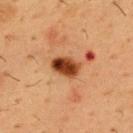| field | value |
|---|---|
| workup | imaged on a skin check; not biopsied |
| automated lesion analysis | a footprint of about 7 mm²; a lesion–skin lightness drop of about 17 and a normalized border contrast of about 13 |
| size | ≈3.5 mm |
| acquisition | ~15 mm tile from a whole-body skin photo |
| tile lighting | cross-polarized illumination |
| subject | male, roughly 55 years of age |
| body site | the chest |